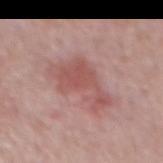The lesion was tiled from a total-body skin photograph and was not biopsied.
Longest diameter approximately 5.5 mm.
The patient is a male roughly 50 years of age.
This is a white-light tile.
Cropped from a whole-body photographic skin survey; the tile spans about 15 mm.
Automated image analysis of the tile measured an area of roughly 11 mm² and a symmetry-axis asymmetry near 0.7. The analysis additionally found a lesion–skin lightness drop of about 9. The analysis additionally found a border-irregularity rating of about 8.5/10, a within-lesion color-variation index near 1.5/10, and a peripheral color-asymmetry measure near 0.5. And it measured an automated nevus-likeness rating near 80 out of 100.
The lesion is located on the back.